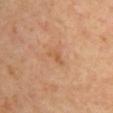Recorded during total-body skin imaging; not selected for excision or biopsy.
Cropped from a total-body skin-imaging series; the visible field is about 15 mm.
The lesion is on the right upper arm.
The patient is a female in their mid- to late 40s.
The recorded lesion diameter is about 2.5 mm.
The lesion-visualizer software estimated an average lesion color of about L≈58 a*≈23 b*≈38 (CIELAB) and a normalized lesion–skin contrast near 5. The analysis additionally found lesion-presence confidence of about 100/100.
Captured under cross-polarized illumination.